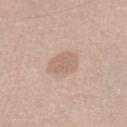Case summary:
- lesion diameter — ≈3.5 mm
- illumination — white-light illumination
- patient — male, in their 60s
- image — total-body-photography crop, ~15 mm field of view
- TBP lesion metrics — a lesion area of about 7.5 mm² and an eccentricity of roughly 0.6
- site — the right lower leg